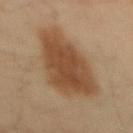Recorded during total-body skin imaging; not selected for excision or biopsy.
The lesion-visualizer software estimated an area of roughly 35 mm² and two-axis asymmetry of about 0.25. And it measured a normalized border contrast of about 9. The analysis additionally found a border-irregularity index near 3/10, a within-lesion color-variation index near 3/10, and radial color variation of about 1. The analysis additionally found a classifier nevus-likeness of about 100/100 and lesion-presence confidence of about 100/100.
A male subject, approximately 35 years of age.
On the mid back.
This is a cross-polarized tile.
Cropped from a total-body skin-imaging series; the visible field is about 15 mm.
Measured at roughly 8.5 mm in maximum diameter.